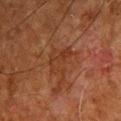This lesion was catalogued during total-body skin photography and was not selected for biopsy. Measured at roughly 4 mm in maximum diameter. A male subject roughly 65 years of age. A roughly 15 mm field-of-view crop from a total-body skin photograph. The lesion is located on the head or neck. This is a cross-polarized tile.